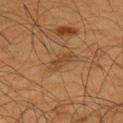Imaged during a routine full-body skin examination; the lesion was not biopsied and no histopathology is available. On the arm. A male patient, aged around 65. A lesion tile, about 15 mm wide, cut from a 3D total-body photograph. The recorded lesion diameter is about 3.5 mm. An algorithmic analysis of the crop reported an area of roughly 4.5 mm², an outline eccentricity of about 0.85 (0 = round, 1 = elongated), and a symmetry-axis asymmetry near 0.3. The software also gave border irregularity of about 3.5 on a 0–10 scale, internal color variation of about 2 on a 0–10 scale, and radial color variation of about 0.5. And it measured a lesion-detection confidence of about 100/100.